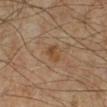biopsy_status: not biopsied; imaged during a skin examination
lesion_size:
  long_diameter_mm_approx: 2.5
lighting: cross-polarized
patient:
  sex: male
  age_approx: 60
automated_metrics:
  eccentricity: 0.65
  shape_asymmetry: 0.25
  lesion_detection_confidence_0_100: 100
site: left lower leg
image:
  source: total-body photography crop
  field_of_view_mm: 15